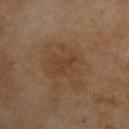diameter = ≈3 mm | automated metrics = a lesion–skin lightness drop of about 5; a border-irregularity rating of about 7/10 and a color-variation rating of about 0/10; a nevus-likeness score of about 0/100 and a detector confidence of about 100 out of 100 that the crop contains a lesion | subject = male, in their mid- to late 50s | imaging modality = ~15 mm crop, total-body skin-cancer survey | anatomic site = the upper back.